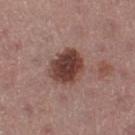biopsy_status: not biopsied; imaged during a skin examination
image:
  source: total-body photography crop
  field_of_view_mm: 15
site: left thigh
patient:
  sex: female
  age_approx: 55